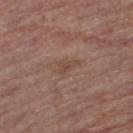<record>
<biopsy_status>not biopsied; imaged during a skin examination</biopsy_status>
<lesion_size>
  <long_diameter_mm_approx>2.5</long_diameter_mm_approx>
</lesion_size>
<site>leg</site>
<image>
  <source>total-body photography crop</source>
  <field_of_view_mm>15</field_of_view_mm>
</image>
<patient>
  <sex>male</sex>
  <age_approx>55</age_approx>
</patient>
</record>